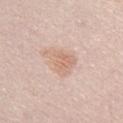biopsy_status: not biopsied; imaged during a skin examination
image:
  source: total-body photography crop
  field_of_view_mm: 15
lighting: white-light
patient:
  sex: female
  age_approx: 60
site: chest